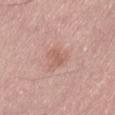{"biopsy_status": "not biopsied; imaged during a skin examination", "image": {"source": "total-body photography crop", "field_of_view_mm": 15}, "patient": {"sex": "male", "age_approx": 70}, "lighting": "white-light", "site": "lower back"}